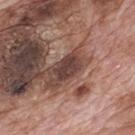Q: Is there a histopathology result?
A: imaged on a skin check; not biopsied